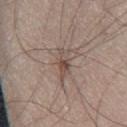No biopsy was performed on this lesion — it was imaged during a full skin examination and was not determined to be concerning. From the leg. A male patient aged 43 to 47. Automated image analysis of the tile measured a mean CIELAB color near L≈49 a*≈15 b*≈22, roughly 9 lightness units darker than nearby skin, and a normalized border contrast of about 6.5. It also reported a border-irregularity rating of about 5/10, internal color variation of about 4 on a 0–10 scale, and a peripheral color-asymmetry measure near 1. And it measured a lesion-detection confidence of about 100/100. A close-up tile cropped from a whole-body skin photograph, about 15 mm across. This is a white-light tile. The lesion's longest dimension is about 4 mm.